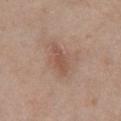Assessment:
Recorded during total-body skin imaging; not selected for excision or biopsy.
Image and clinical context:
From the chest. A female patient, aged approximately 40. A close-up tile cropped from a whole-body skin photograph, about 15 mm across.